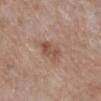Q: Is there a histopathology result?
A: imaged on a skin check; not biopsied
Q: What are the patient's age and sex?
A: female, aged approximately 55
Q: Lesion size?
A: ≈3.5 mm
Q: What is the anatomic site?
A: the leg
Q: What kind of image is this?
A: ~15 mm tile from a whole-body skin photo